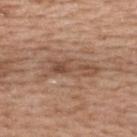No biopsy was performed on this lesion — it was imaged during a full skin examination and was not determined to be concerning. Approximately 7 mm at its widest. A close-up tile cropped from a whole-body skin photograph, about 15 mm across. Automated image analysis of the tile measured an outline eccentricity of about 0.95 (0 = round, 1 = elongated) and a symmetry-axis asymmetry near 0.25. It also reported a mean CIELAB color near L≈51 a*≈19 b*≈29, about 9 CIELAB-L* units darker than the surrounding skin, and a lesion-to-skin contrast of about 6.5 (normalized; higher = more distinct). The software also gave a border-irregularity index near 4.5/10, a color-variation rating of about 6/10, and a peripheral color-asymmetry measure near 2.5. The lesion is located on the upper back. Imaged with white-light lighting. The subject is a female approximately 60 years of age.